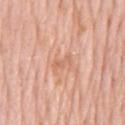site: the chest
tile lighting: white-light illumination
image source: ~15 mm tile from a whole-body skin photo
subject: male, in their 80s